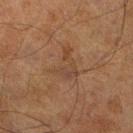Captured during whole-body skin photography for melanoma surveillance; the lesion was not biopsied. The recorded lesion diameter is about 3.5 mm. A lesion tile, about 15 mm wide, cut from a 3D total-body photograph. Captured under cross-polarized illumination. The lesion is located on the right lower leg. The total-body-photography lesion software estimated a lesion area of about 6.5 mm² and an outline eccentricity of about 0.75 (0 = round, 1 = elongated). The analysis additionally found a mean CIELAB color near L≈35 a*≈16 b*≈26, about 5 CIELAB-L* units darker than the surrounding skin, and a normalized border contrast of about 5. The analysis additionally found a border-irregularity rating of about 7.5/10, a color-variation rating of about 2.5/10, and radial color variation of about 1. And it measured a classifier nevus-likeness of about 0/100 and a lesion-detection confidence of about 100/100. A male patient aged 68–72.